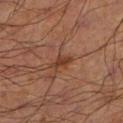follow-up — imaged on a skin check; not biopsied
body site — the left lower leg
image — total-body-photography crop, ~15 mm field of view
subject — male, roughly 70 years of age
lighting — cross-polarized
size — about 2.5 mm